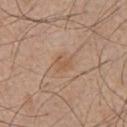biopsy status = imaged on a skin check; not biopsied
body site = the chest
patient = male, aged approximately 55
image = ~15 mm tile from a whole-body skin photo
illumination = white-light